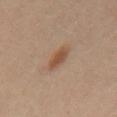Impression:
This lesion was catalogued during total-body skin photography and was not selected for biopsy.
Context:
The patient is a male aged 38 to 42. About 3.5 mm across. Imaged with cross-polarized lighting. Cropped from a whole-body photographic skin survey; the tile spans about 15 mm. On the abdomen.